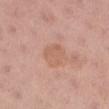| feature | finding |
|---|---|
| follow-up | catalogued during a skin exam; not biopsied |
| patient | female, aged 53–57 |
| imaging modality | ~15 mm tile from a whole-body skin photo |
| body site | the left lower leg |